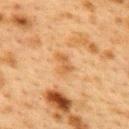<record>
<biopsy_status>not biopsied; imaged during a skin examination</biopsy_status>
<lighting>cross-polarized</lighting>
<patient>
  <sex>female</sex>
  <age_approx>40</age_approx>
</patient>
<image>
  <source>total-body photography crop</source>
  <field_of_view_mm>15</field_of_view_mm>
</image>
<lesion_size>
  <long_diameter_mm_approx>2.5</long_diameter_mm_approx>
</lesion_size>
<site>upper back</site>
</record>